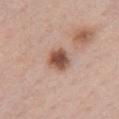Assessment: Imaged during a routine full-body skin examination; the lesion was not biopsied and no histopathology is available. Acquisition and patient details: Automated image analysis of the tile measured a border-irregularity rating of about 2/10, internal color variation of about 4.5 on a 0–10 scale, and a peripheral color-asymmetry measure near 1.5. The analysis additionally found a detector confidence of about 100 out of 100 that the crop contains a lesion. This is a white-light tile. The lesion is located on the chest. Cropped from a whole-body photographic skin survey; the tile spans about 15 mm. A female patient, aged around 40.